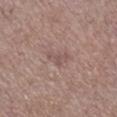Recorded during total-body skin imaging; not selected for excision or biopsy.
A female subject, roughly 65 years of age.
The lesion's longest dimension is about 3 mm.
The tile uses white-light illumination.
A lesion tile, about 15 mm wide, cut from a 3D total-body photograph.
From the right lower leg.